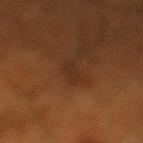| feature | finding |
|---|---|
| notes | imaged on a skin check; not biopsied |
| site | the left lower leg |
| imaging modality | 15 mm crop, total-body photography |
| subject | male, approximately 60 years of age |
| illumination | cross-polarized |
| size | ≈3 mm |